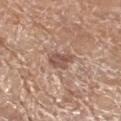• workup — catalogued during a skin exam; not biopsied
• size — about 2.5 mm
• tile lighting — white-light
• imaging modality — ~15 mm crop, total-body skin-cancer survey
• automated metrics — a border-irregularity index near 3.5/10 and a within-lesion color-variation index near 3/10
• anatomic site — the left lower leg
• patient — female, approximately 75 years of age